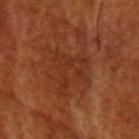Assessment:
No biopsy was performed on this lesion — it was imaged during a full skin examination and was not determined to be concerning.
Clinical summary:
A region of skin cropped from a whole-body photographic capture, roughly 15 mm wide. The lesion-visualizer software estimated a mean CIELAB color near L≈23 a*≈20 b*≈25, about 4 CIELAB-L* units darker than the surrounding skin, and a lesion-to-skin contrast of about 5 (normalized; higher = more distinct). The lesion is on the head or neck. A female subject, about 80 years old.